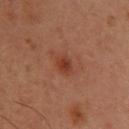On the head or neck. This image is a 15 mm lesion crop taken from a total-body photograph. Imaged with cross-polarized lighting. A female patient, approximately 40 years of age. The lesion's longest dimension is about 2.5 mm. Automated image analysis of the tile measured a border-irregularity rating of about 2/10 and internal color variation of about 2.5 on a 0–10 scale.